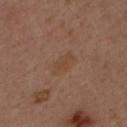Case summary:
• biopsy status · total-body-photography surveillance lesion; no biopsy
• site · the upper back
• patient · male, aged approximately 35
• acquisition · ~15 mm crop, total-body skin-cancer survey